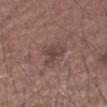workup: total-body-photography surveillance lesion; no biopsy | image-analysis metrics: an area of roughly 5 mm², an outline eccentricity of about 0.8 (0 = round, 1 = elongated), and a shape-asymmetry score of about 0.45 (0 = symmetric) | acquisition: ~15 mm crop, total-body skin-cancer survey | lighting: white-light illumination | patient: male, roughly 65 years of age | body site: the right forearm.